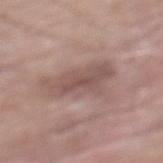Assessment: The lesion was photographed on a routine skin check and not biopsied; there is no pathology result. Background: A male subject, about 60 years old. The tile uses white-light illumination. The lesion's longest dimension is about 6 mm. A 15 mm crop from a total-body photograph taken for skin-cancer surveillance. From the mid back.